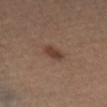Q: Was this lesion biopsied?
A: no biopsy performed (imaged during a skin exam)
Q: How was the tile lit?
A: cross-polarized
Q: What is the imaging modality?
A: total-body-photography crop, ~15 mm field of view
Q: What is the lesion's diameter?
A: ≈2.5 mm
Q: What are the patient's age and sex?
A: male, aged 48 to 52
Q: What is the anatomic site?
A: the left leg
Q: What did automated image analysis measure?
A: a shape-asymmetry score of about 0.15 (0 = symmetric); an average lesion color of about L≈38 a*≈18 b*≈25 (CIELAB) and a normalized lesion–skin contrast near 7.5; border irregularity of about 1.5 on a 0–10 scale and a color-variation rating of about 2/10; a nevus-likeness score of about 85/100 and lesion-presence confidence of about 100/100